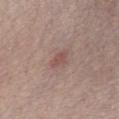<case>
<biopsy_status>not biopsied; imaged during a skin examination</biopsy_status>
<patient>
  <sex>male</sex>
  <age_approx>30</age_approx>
</patient>
<lesion_size>
  <long_diameter_mm_approx>2.5</long_diameter_mm_approx>
</lesion_size>
<image>
  <source>total-body photography crop</source>
  <field_of_view_mm>15</field_of_view_mm>
</image>
<automated_metrics>
  <cielab_L>51</cielab_L>
  <cielab_a>20</cielab_a>
  <cielab_b>21</cielab_b>
  <border_irregularity_0_10>2.5</border_irregularity_0_10>
  <color_variation_0_10>0.5</color_variation_0_10>
  <peripheral_color_asymmetry>0.0</peripheral_color_asymmetry>
</automated_metrics>
<lighting>white-light</lighting>
</case>